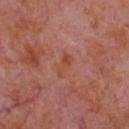Clinical impression:
Recorded during total-body skin imaging; not selected for excision or biopsy.
Context:
Automated tile analysis of the lesion measured an automated nevus-likeness rating near 0 out of 100. Located on the chest. The tile uses white-light illumination. Approximately 3 mm at its widest. A male subject, aged approximately 65. A region of skin cropped from a whole-body photographic capture, roughly 15 mm wide.